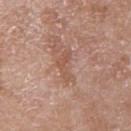{
  "biopsy_status": "not biopsied; imaged during a skin examination",
  "lighting": "white-light",
  "site": "left upper arm",
  "image": {
    "source": "total-body photography crop",
    "field_of_view_mm": 15
  },
  "patient": {
    "sex": "female",
    "age_approx": 70
  },
  "lesion_size": {
    "long_diameter_mm_approx": 4.0
  }
}